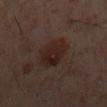Recorded during total-body skin imaging; not selected for excision or biopsy. The lesion's longest dimension is about 4.5 mm. A close-up tile cropped from a whole-body skin photograph, about 15 mm across. Captured under cross-polarized illumination. A male subject aged approximately 60. On the mid back.